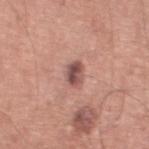<lesion>
<biopsy_status>not biopsied; imaged during a skin examination</biopsy_status>
<automated_metrics>
  <cielab_L>51</cielab_L>
  <cielab_a>22</cielab_a>
  <cielab_b>23</cielab_b>
  <vs_skin_darker_L>13.0</vs_skin_darker_L>
</automated_metrics>
<site>left thigh</site>
<patient>
  <sex>male</sex>
  <age_approx>70</age_approx>
</patient>
<image>
  <source>total-body photography crop</source>
  <field_of_view_mm>15</field_of_view_mm>
</image>
<lighting>white-light</lighting>
</lesion>